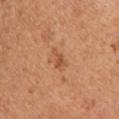The lesion was tiled from a total-body skin photograph and was not biopsied. The lesion-visualizer software estimated a mean CIELAB color near L≈53 a*≈25 b*≈36 and about 9 CIELAB-L* units darker than the surrounding skin. The software also gave a classifier nevus-likeness of about 5/100. A female subject, approximately 45 years of age. From the upper back. A 15 mm close-up tile from a total-body photography series done for melanoma screening.